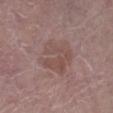| key | value |
|---|---|
| biopsy status | total-body-photography surveillance lesion; no biopsy |
| lesion size | about 4.5 mm |
| automated lesion analysis | a lesion area of about 15 mm², an eccentricity of roughly 0.4, and a symmetry-axis asymmetry near 0.3 |
| acquisition | total-body-photography crop, ~15 mm field of view |
| lighting | white-light illumination |
| patient | male, aged around 65 |
| site | the right lower leg |